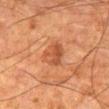biopsy_status: not biopsied; imaged during a skin examination
image:
  source: total-body photography crop
  field_of_view_mm: 15
site: right thigh
patient:
  sex: male
  age_approx: 80
automated_metrics:
  border_irregularity_0_10: 2.5
  color_variation_0_10: 4.0
  peripheral_color_asymmetry: 1.5
lesion_size:
  long_diameter_mm_approx: 3.5